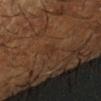biopsy status: imaged on a skin check; not biopsied
TBP lesion metrics: a footprint of about 2.5 mm² and a symmetry-axis asymmetry near 0.35; a border-irregularity index near 3.5/10, a within-lesion color-variation index near 0.5/10, and peripheral color asymmetry of about 0; a nevus-likeness score of about 0/100 and a lesion-detection confidence of about 85/100
location: the arm
size: ~2.5 mm (longest diameter)
acquisition: 15 mm crop, total-body photography
tile lighting: cross-polarized illumination
subject: male, aged around 65